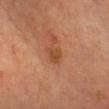Part of a total-body skin-imaging series; this lesion was reviewed on a skin check and was not flagged for biopsy. A 15 mm crop from a total-body photograph taken for skin-cancer surveillance. A female patient, about 40 years old. The lesion is on the chest.